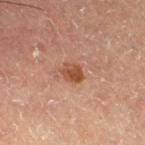  biopsy_status: not biopsied; imaged during a skin examination
  automated_metrics:
    area_mm2_approx: 4.5
    eccentricity: 0.6
    shape_asymmetry: 0.2
    cielab_L: 43
    cielab_a: 22
    cielab_b: 30
    nevus_likeness_0_100: 75
    lesion_detection_confidence_0_100: 100
  site: right thigh
  patient:
    sex: male
    age_approx: 60
  image:
    source: total-body photography crop
    field_of_view_mm: 15
  lesion_size:
    long_diameter_mm_approx: 2.5
  lighting: cross-polarized